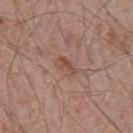Q: Was this lesion biopsied?
A: catalogued during a skin exam; not biopsied
Q: Patient demographics?
A: male, aged 68 to 72
Q: What is the lesion's diameter?
A: ~3 mm (longest diameter)
Q: Automated lesion metrics?
A: border irregularity of about 2.5 on a 0–10 scale, internal color variation of about 3 on a 0–10 scale, and peripheral color asymmetry of about 1
Q: Illumination type?
A: white-light
Q: What is the anatomic site?
A: the chest
Q: What is the imaging modality?
A: 15 mm crop, total-body photography